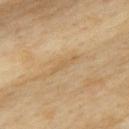The lesion was tiled from a total-body skin photograph and was not biopsied. From the upper back. A female subject roughly 60 years of age. A region of skin cropped from a whole-body photographic capture, roughly 15 mm wide. The total-body-photography lesion software estimated an outline eccentricity of about 0.95 (0 = round, 1 = elongated) and a shape-asymmetry score of about 0.5 (0 = symmetric). The software also gave a lesion color around L≈54 a*≈14 b*≈36 in CIELAB, about 6 CIELAB-L* units darker than the surrounding skin, and a normalized lesion–skin contrast near 5. This is a cross-polarized tile.